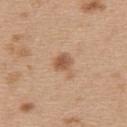This lesion was catalogued during total-body skin photography and was not selected for biopsy.
The recorded lesion diameter is about 3 mm.
The subject is a female aged 43 to 47.
On the upper back.
A 15 mm close-up extracted from a 3D total-body photography capture.